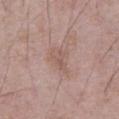workup: total-body-photography surveillance lesion; no biopsy
subject: male, aged around 65
site: the abdomen
illumination: white-light
TBP lesion metrics: a footprint of about 3 mm², an eccentricity of roughly 0.75, and two-axis asymmetry of about 0.45; a lesion color around L≈55 a*≈18 b*≈24 in CIELAB, roughly 7 lightness units darker than nearby skin, and a normalized lesion–skin contrast near 5; a border-irregularity index near 4.5/10, internal color variation of about 0 on a 0–10 scale, and peripheral color asymmetry of about 0; a classifier nevus-likeness of about 0/100 and a detector confidence of about 100 out of 100 that the crop contains a lesion
imaging modality: total-body-photography crop, ~15 mm field of view